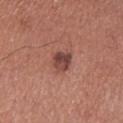follow-up — no biopsy performed (imaged during a skin exam) | illumination — white-light | subject — male, aged approximately 70 | site — the left lower leg | image-analysis metrics — a shape eccentricity near 0.35 and a shape-asymmetry score of about 0.25 (0 = symmetric); a mean CIELAB color near L≈44 a*≈23 b*≈23 and a normalized border contrast of about 9; a classifier nevus-likeness of about 80/100 and lesion-presence confidence of about 100/100 | lesion diameter — ≈2.5 mm | acquisition — 15 mm crop, total-body photography.